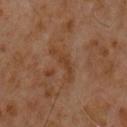Imaged with cross-polarized lighting.
Located on the chest.
A male patient, approximately 60 years of age.
A 15 mm close-up extracted from a 3D total-body photography capture.
The lesion's longest dimension is about 4.5 mm.